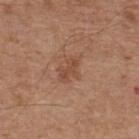Impression: This lesion was catalogued during total-body skin photography and was not selected for biopsy. Acquisition and patient details: A close-up tile cropped from a whole-body skin photograph, about 15 mm across. A male patient, in their mid- to late 60s. The tile uses white-light illumination. The recorded lesion diameter is about 3.5 mm. The total-body-photography lesion software estimated a nevus-likeness score of about 0/100 and a detector confidence of about 100 out of 100 that the crop contains a lesion. The lesion is located on the upper back.